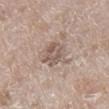{
  "biopsy_status": "not biopsied; imaged during a skin examination",
  "patient": {
    "sex": "female",
    "age_approx": 75
  },
  "image": {
    "source": "total-body photography crop",
    "field_of_view_mm": 15
  },
  "automated_metrics": {
    "area_mm2_approx": 9.0,
    "shape_asymmetry": 0.25,
    "vs_skin_darker_L": 10.0,
    "vs_skin_contrast_norm": 6.5
  },
  "site": "right lower leg"
}